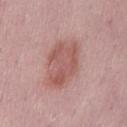workup = imaged on a skin check; not biopsied | site = the lower back | diameter = ~6 mm (longest diameter) | image source = total-body-photography crop, ~15 mm field of view | automated metrics = an area of roughly 16 mm² and an eccentricity of roughly 0.85; a classifier nevus-likeness of about 70/100 | patient = male, about 55 years old.